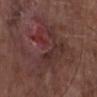Assessment: The lesion was tiled from a total-body skin photograph and was not biopsied. Background: Located on the upper back. A close-up tile cropped from a whole-body skin photograph, about 15 mm across. Captured under white-light illumination. About 8.5 mm across. The patient is a male about 50 years old.